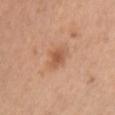The lesion was tiled from a total-body skin photograph and was not biopsied. The lesion is located on the chest. The patient is a female approximately 30 years of age. A 15 mm close-up extracted from a 3D total-body photography capture.